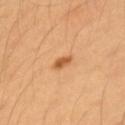size = ~2 mm (longest diameter)
body site = the left upper arm
illumination = cross-polarized illumination
subject = female, in their 40s
imaging modality = ~15 mm crop, total-body skin-cancer survey